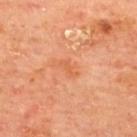The lesion was tiled from a total-body skin photograph and was not biopsied. The lesion is on the upper back. Automated image analysis of the tile measured a footprint of about 2.5 mm², an outline eccentricity of about 0.85 (0 = round, 1 = elongated), and two-axis asymmetry of about 0.5. And it measured a nevus-likeness score of about 0/100. About 2.5 mm across. Imaged with cross-polarized lighting. A male patient, approximately 65 years of age. A lesion tile, about 15 mm wide, cut from a 3D total-body photograph.